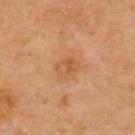Q: Where on the body is the lesion?
A: the upper back
Q: Automated lesion metrics?
A: a border-irregularity rating of about 2/10, a within-lesion color-variation index near 4.5/10, and radial color variation of about 1.5; a nevus-likeness score of about 0/100 and a detector confidence of about 100 out of 100 that the crop contains a lesion
Q: What lighting was used for the tile?
A: cross-polarized
Q: What kind of image is this?
A: 15 mm crop, total-body photography
Q: Who is the patient?
A: male, in their 70s